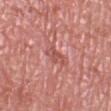Impression: This lesion was catalogued during total-body skin photography and was not selected for biopsy. Clinical summary: The lesion's longest dimension is about 2.5 mm. The lesion is on the leg. A 15 mm crop from a total-body photograph taken for skin-cancer surveillance. The subject is a female in their mid- to late 50s. Imaged with white-light lighting.